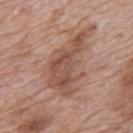{"biopsy_status": "not biopsied; imaged during a skin examination", "lighting": "white-light", "patient": {"sex": "male", "age_approx": 70}, "image": {"source": "total-body photography crop", "field_of_view_mm": 15}, "lesion_size": {"long_diameter_mm_approx": 8.0}, "site": "back"}